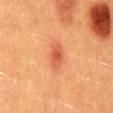Q: Is there a histopathology result?
A: catalogued during a skin exam; not biopsied
Q: What did automated image analysis measure?
A: a lesion color around L≈48 a*≈28 b*≈34 in CIELAB, roughly 9 lightness units darker than nearby skin, and a lesion-to-skin contrast of about 6.5 (normalized; higher = more distinct); a classifier nevus-likeness of about 65/100 and a lesion-detection confidence of about 100/100
Q: What are the patient's age and sex?
A: male, roughly 40 years of age
Q: Lesion location?
A: the mid back
Q: What is the imaging modality?
A: ~15 mm tile from a whole-body skin photo
Q: Illumination type?
A: cross-polarized illumination
Q: What is the lesion's diameter?
A: ~3.5 mm (longest diameter)